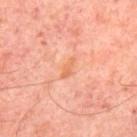| key | value |
|---|---|
| follow-up | catalogued during a skin exam; not biopsied |
| lesion diameter | ≈2.5 mm |
| site | the upper back |
| patient | aged 53–57 |
| acquisition | ~15 mm tile from a whole-body skin photo |
| illumination | cross-polarized illumination |